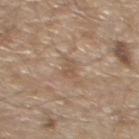workup=total-body-photography surveillance lesion; no biopsy
site=the front of the torso
acquisition=total-body-photography crop, ~15 mm field of view
subject=male, in their 70s